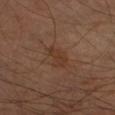No biopsy was performed on this lesion — it was imaged during a full skin examination and was not determined to be concerning. A male patient roughly 70 years of age. An algorithmic analysis of the crop reported a symmetry-axis asymmetry near 0.25. The software also gave a lesion color around L≈33 a*≈19 b*≈27 in CIELAB and roughly 5 lightness units darker than nearby skin. And it measured an automated nevus-likeness rating near 0 out of 100 and a detector confidence of about 100 out of 100 that the crop contains a lesion. A lesion tile, about 15 mm wide, cut from a 3D total-body photograph. The lesion is on the right forearm. About 3 mm across.